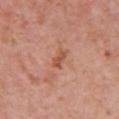| key | value |
|---|---|
| lesion diameter | about 2.5 mm |
| body site | the chest |
| subject | female, aged 38 to 42 |
| imaging modality | 15 mm crop, total-body photography |
| tile lighting | white-light illumination |